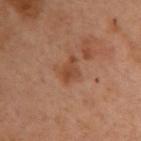  biopsy_status: not biopsied; imaged during a skin examination
  image:
    source: total-body photography crop
    field_of_view_mm: 15
  automated_metrics:
    area_mm2_approx: 5.0
    cielab_L: 37
    cielab_a: 19
    cielab_b: 28
    vs_skin_darker_L: 7.0
    vs_skin_contrast_norm: 6.5
  site: upper back
  lesion_size:
    long_diameter_mm_approx: 3.0
  patient:
    sex: female
    age_approx: 55